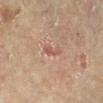Case summary:
– patient — female, roughly 80 years of age
– tile lighting — cross-polarized
– size — about 3 mm
– imaging modality — 15 mm crop, total-body photography
– body site — the right lower leg